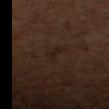Clinical impression: Recorded during total-body skin imaging; not selected for excision or biopsy. Context: Longest diameter approximately 3 mm. Automated image analysis of the tile measured a lesion area of about 3.5 mm², a shape eccentricity near 0.85, and two-axis asymmetry of about 0.5. It also reported an automated nevus-likeness rating near 0 out of 100 and lesion-presence confidence of about 100/100. Imaged with cross-polarized lighting. The subject is a male aged around 65. A 15 mm crop from a total-body photograph taken for skin-cancer surveillance.